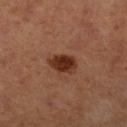Q: What did automated image analysis measure?
A: a lesion color around L≈33 a*≈25 b*≈30 in CIELAB and a normalized lesion–skin contrast near 11.5; a detector confidence of about 100 out of 100 that the crop contains a lesion
Q: What lighting was used for the tile?
A: cross-polarized
Q: What are the patient's age and sex?
A: female
Q: How large is the lesion?
A: ~3.5 mm (longest diameter)
Q: Where on the body is the lesion?
A: the right lower leg
Q: What kind of image is this?
A: ~15 mm tile from a whole-body skin photo